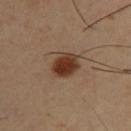{"biopsy_status": "not biopsied; imaged during a skin examination", "automated_metrics": {"eccentricity": 0.6, "shape_asymmetry": 0.2, "border_irregularity_0_10": 2.0, "color_variation_0_10": 4.5, "peripheral_color_asymmetry": 1.5}, "lesion_size": {"long_diameter_mm_approx": 3.5}, "patient": {"sex": "male", "age_approx": 40}, "site": "back", "lighting": "cross-polarized", "image": {"source": "total-body photography crop", "field_of_view_mm": 15}}